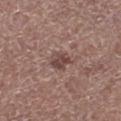Clinical impression:
The lesion was photographed on a routine skin check and not biopsied; there is no pathology result.
Clinical summary:
A male subject, roughly 65 years of age. On the right lower leg. An algorithmic analysis of the crop reported a mean CIELAB color near L≈44 a*≈19 b*≈21. The software also gave a within-lesion color-variation index near 3/10 and a peripheral color-asymmetry measure near 1. A lesion tile, about 15 mm wide, cut from a 3D total-body photograph.